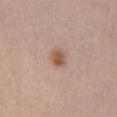Clinical impression:
Captured during whole-body skin photography for melanoma surveillance; the lesion was not biopsied.
Image and clinical context:
A female patient, aged approximately 65. On the chest. Captured under white-light illumination. A 15 mm crop from a total-body photograph taken for skin-cancer surveillance.